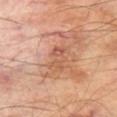follow-up: no biopsy performed (imaged during a skin exam) | location: the left thigh | tile lighting: cross-polarized illumination | subject: male, about 70 years old | image: ~15 mm crop, total-body skin-cancer survey.